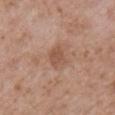No biopsy was performed on this lesion — it was imaged during a full skin examination and was not determined to be concerning. Imaged with white-light lighting. A male patient, roughly 50 years of age. The lesion is located on the front of the torso. A 15 mm crop from a total-body photograph taken for skin-cancer surveillance. An algorithmic analysis of the crop reported an area of roughly 5 mm² and a shape eccentricity near 0.6. It also reported border irregularity of about 1.5 on a 0–10 scale, a color-variation rating of about 1.5/10, and peripheral color asymmetry of about 0.5. It also reported a nevus-likeness score of about 0/100. The lesion's longest dimension is about 2.5 mm.